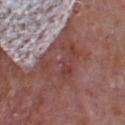workup = catalogued during a skin exam; not biopsied
patient = male, aged approximately 65
image source = total-body-photography crop, ~15 mm field of view
site = the front of the torso